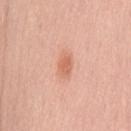{
  "biopsy_status": "not biopsied; imaged during a skin examination",
  "lesion_size": {
    "long_diameter_mm_approx": 2.5
  },
  "patient": {
    "sex": "female",
    "age_approx": 50
  },
  "site": "mid back",
  "lighting": "white-light",
  "image": {
    "source": "total-body photography crop",
    "field_of_view_mm": 15
  }
}